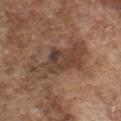Notes:
• tile lighting: white-light illumination
• patient: male, roughly 75 years of age
• location: the chest
• imaging modality: ~15 mm tile from a whole-body skin photo